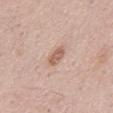Context: A male patient aged 53–57. Automated tile analysis of the lesion measured a lesion area of about 3.5 mm², a shape eccentricity near 0.8, and a shape-asymmetry score of about 0.2 (0 = symmetric). The software also gave an average lesion color of about L≈60 a*≈20 b*≈28 (CIELAB), roughly 11 lightness units darker than nearby skin, and a normalized border contrast of about 7.5. The software also gave a border-irregularity rating of about 2/10 and a peripheral color-asymmetry measure near 1. It also reported a nevus-likeness score of about 60/100. A region of skin cropped from a whole-body photographic capture, roughly 15 mm wide. Measured at roughly 2.5 mm in maximum diameter. Located on the front of the torso. The tile uses white-light illumination.